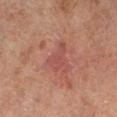Q: Was a biopsy performed?
A: imaged on a skin check; not biopsied
Q: How was this image acquired?
A: ~15 mm tile from a whole-body skin photo
Q: What is the anatomic site?
A: the leg
Q: Who is the patient?
A: male, aged 68 to 72